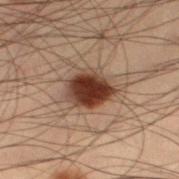workup = total-body-photography surveillance lesion; no biopsy | location = the leg | acquisition = 15 mm crop, total-body photography | tile lighting = cross-polarized illumination | diameter = ~4.5 mm (longest diameter) | subject = male, aged approximately 55.